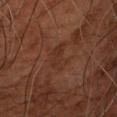Clinical impression: Captured during whole-body skin photography for melanoma surveillance; the lesion was not biopsied. Image and clinical context: This image is a 15 mm lesion crop taken from a total-body photograph. The total-body-photography lesion software estimated an area of roughly 5 mm², an outline eccentricity of about 0.85 (0 = round, 1 = elongated), and a symmetry-axis asymmetry near 0.35. The software also gave a lesion color around L≈28 a*≈20 b*≈26 in CIELAB, roughly 5 lightness units darker than nearby skin, and a normalized lesion–skin contrast near 5. From the upper back. The subject is a male aged 53–57. Approximately 3.5 mm at its widest.